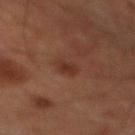Q: Was this lesion biopsied?
A: catalogued during a skin exam; not biopsied
Q: What is the imaging modality?
A: ~15 mm crop, total-body skin-cancer survey
Q: Lesion size?
A: ≈3 mm
Q: How was the tile lit?
A: cross-polarized illumination
Q: What are the patient's age and sex?
A: male, aged 58 to 62
Q: Lesion location?
A: the chest